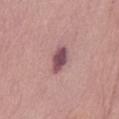Impression: Recorded during total-body skin imaging; not selected for excision or biopsy. Image and clinical context: The patient is a female in their mid-80s or older. A roughly 15 mm field-of-view crop from a total-body skin photograph. The lesion-visualizer software estimated an eccentricity of roughly 0.85. The software also gave a lesion color around L≈50 a*≈24 b*≈15 in CIELAB, about 15 CIELAB-L* units darker than the surrounding skin, and a lesion-to-skin contrast of about 11 (normalized; higher = more distinct). And it measured a border-irregularity index near 2/10, a color-variation rating of about 3/10, and a peripheral color-asymmetry measure near 1. The lesion is located on the abdomen. Imaged with white-light lighting.